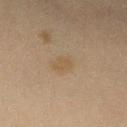follow-up: imaged on a skin check; not biopsied
patient: female, aged around 40
location: the right forearm
automated metrics: an eccentricity of roughly 0.75 and a shape-asymmetry score of about 0.25 (0 = symmetric); about 4 CIELAB-L* units darker than the surrounding skin
illumination: cross-polarized
image source: ~15 mm crop, total-body skin-cancer survey